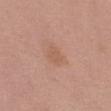workup: catalogued during a skin exam; not biopsied
subject: female, about 65 years old
diameter: about 3.5 mm
imaging modality: ~15 mm tile from a whole-body skin photo
image-analysis metrics: a lesion-detection confidence of about 100/100
body site: the left thigh
illumination: white-light illumination